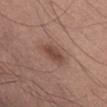* follow-up — catalogued during a skin exam; not biopsied
* imaging modality — ~15 mm tile from a whole-body skin photo
* automated metrics — a footprint of about 5.5 mm², an eccentricity of roughly 0.8, and two-axis asymmetry of about 0.25; a lesion color around L≈45 a*≈20 b*≈25 in CIELAB and a lesion-to-skin contrast of about 7.5 (normalized; higher = more distinct); a nevus-likeness score of about 60/100 and a lesion-detection confidence of about 100/100
* lesion diameter — ≈3.5 mm
* tile lighting — white-light illumination
* patient — male, aged 53–57
* location — the abdomen